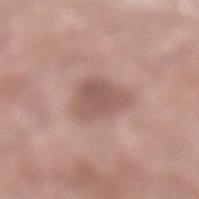Captured during whole-body skin photography for melanoma surveillance; the lesion was not biopsied. Imaged with white-light lighting. The patient is a male aged 63–67. Automated tile analysis of the lesion measured a nevus-likeness score of about 5/100 and a lesion-detection confidence of about 100/100. A 15 mm close-up extracted from a 3D total-body photography capture. The lesion is located on the left lower leg.